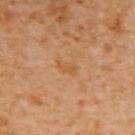Assessment: This lesion was catalogued during total-body skin photography and was not selected for biopsy. Context: An algorithmic analysis of the crop reported border irregularity of about 4 on a 0–10 scale and a peripheral color-asymmetry measure near 0. It also reported a classifier nevus-likeness of about 0/100. A 15 mm crop from a total-body photograph taken for skin-cancer surveillance. The lesion is located on the upper back. A female patient, in their mid- to late 50s. Approximately 2.5 mm at its widest.